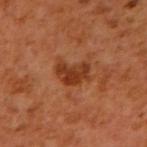Findings:
- anatomic site: the right upper arm
- image source: ~15 mm crop, total-body skin-cancer survey
- subject: male, about 60 years old